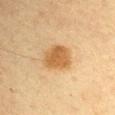Case summary:
* follow-up: no biopsy performed (imaged during a skin exam)
* image source: total-body-photography crop, ~15 mm field of view
* patient: male, aged around 60
* lesion diameter: ~3.5 mm (longest diameter)
* anatomic site: the left upper arm
* lighting: cross-polarized
* automated metrics: an area of roughly 9.5 mm², a shape eccentricity near 0.45, and two-axis asymmetry of about 0.2; a mean CIELAB color near L≈53 a*≈19 b*≈39, about 11 CIELAB-L* units darker than the surrounding skin, and a normalized lesion–skin contrast near 8.5